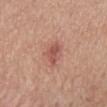  biopsy_status: not biopsied; imaged during a skin examination
  automated_metrics:
    vs_skin_darker_L: 10.0
    vs_skin_contrast_norm: 7.0
    nevus_likeness_0_100: 65
    lesion_detection_confidence_0_100: 100
  lesion_size:
    long_diameter_mm_approx: 2.5
  site: mid back
  patient:
    sex: male
    age_approx: 55
  image:
    source: total-body photography crop
    field_of_view_mm: 15
  lighting: white-light